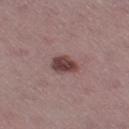Imaged during a routine full-body skin examination; the lesion was not biopsied and no histopathology is available. A male patient in their 40s. The lesion's longest dimension is about 3 mm. Automated image analysis of the tile measured a footprint of about 6 mm² and a symmetry-axis asymmetry near 0.15. It also reported a border-irregularity rating of about 1.5/10 and internal color variation of about 3.5 on a 0–10 scale. Captured under white-light illumination. Located on the left thigh. A lesion tile, about 15 mm wide, cut from a 3D total-body photograph.